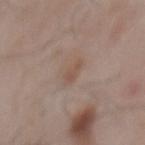Q: Is there a histopathology result?
A: catalogued during a skin exam; not biopsied
Q: Patient demographics?
A: male, roughly 55 years of age
Q: What did automated image analysis measure?
A: a lesion area of about 3.5 mm², a shape eccentricity near 0.85, and a shape-asymmetry score of about 0.35 (0 = symmetric); a mean CIELAB color near L≈52 a*≈16 b*≈25, a lesion–skin lightness drop of about 6, and a normalized lesion–skin contrast near 5.5; a color-variation rating of about 1/10 and peripheral color asymmetry of about 0.5
Q: Where on the body is the lesion?
A: the mid back
Q: What is the imaging modality?
A: ~15 mm tile from a whole-body skin photo
Q: What is the lesion's diameter?
A: about 3 mm